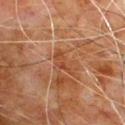Recorded during total-body skin imaging; not selected for excision or biopsy. About 3.5 mm across. Cropped from a total-body skin-imaging series; the visible field is about 15 mm. A male subject, aged approximately 80. The lesion is on the front of the torso.